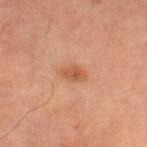The lesion's longest dimension is about 3 mm. Cropped from a total-body skin-imaging series; the visible field is about 15 mm. A female subject, aged 58–62. The total-body-photography lesion software estimated a mean CIELAB color near L≈57 a*≈27 b*≈37 and a lesion–skin lightness drop of about 9. Located on the leg.